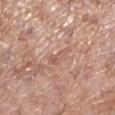Captured during whole-body skin photography for melanoma surveillance; the lesion was not biopsied. The lesion is on the left lower leg. The subject is a female in their mid- to late 70s. About 2.5 mm across. The total-body-photography lesion software estimated a nevus-likeness score of about 0/100. The tile uses white-light illumination. A close-up tile cropped from a whole-body skin photograph, about 15 mm across.